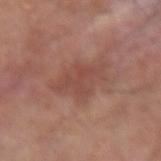Q: Was a biopsy performed?
A: catalogued during a skin exam; not biopsied
Q: Automated lesion metrics?
A: a lesion area of about 14 mm², an outline eccentricity of about 0.7 (0 = round, 1 = elongated), and a symmetry-axis asymmetry near 0.25; a lesion color around L≈48 a*≈23 b*≈26 in CIELAB, about 7 CIELAB-L* units darker than the surrounding skin, and a lesion-to-skin contrast of about 5 (normalized; higher = more distinct)
Q: Who is the patient?
A: male, aged 53 to 57
Q: What is the lesion's diameter?
A: ~5 mm (longest diameter)
Q: What lighting was used for the tile?
A: white-light illumination
Q: Where on the body is the lesion?
A: the right forearm
Q: What kind of image is this?
A: 15 mm crop, total-body photography